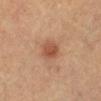size: ~3 mm (longest diameter) | location: the left lower leg | tile lighting: cross-polarized illumination | image source: ~15 mm crop, total-body skin-cancer survey | subject: female, aged around 60.